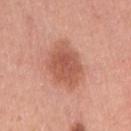Recorded during total-body skin imaging; not selected for excision or biopsy. An algorithmic analysis of the crop reported a mean CIELAB color near L≈56 a*≈27 b*≈31, about 11 CIELAB-L* units darker than the surrounding skin, and a normalized border contrast of about 7.5. The software also gave internal color variation of about 3.5 on a 0–10 scale and a peripheral color-asymmetry measure near 1. A female subject, in their 40s. From the arm. A region of skin cropped from a whole-body photographic capture, roughly 15 mm wide. Longest diameter approximately 4.5 mm. This is a white-light tile.